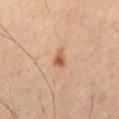Assessment: The lesion was photographed on a routine skin check and not biopsied; there is no pathology result. Clinical summary: A male subject, approximately 60 years of age. Captured under cross-polarized illumination. Measured at roughly 2 mm in maximum diameter. On the abdomen. A lesion tile, about 15 mm wide, cut from a 3D total-body photograph.